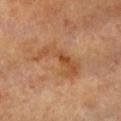lesion size = ~6.5 mm (longest diameter) | illumination = cross-polarized | TBP lesion metrics = a lesion color around L≈52 a*≈23 b*≈38 in CIELAB; a border-irregularity rating of about 7/10 and peripheral color asymmetry of about 1.5 | body site = the upper back | patient = female, aged 73–77 | image source = 15 mm crop, total-body photography.